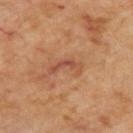Recorded during total-body skin imaging; not selected for excision or biopsy. The lesion-visualizer software estimated a border-irregularity rating of about 7/10, internal color variation of about 0 on a 0–10 scale, and peripheral color asymmetry of about 0. The analysis additionally found lesion-presence confidence of about 100/100. This image is a 15 mm lesion crop taken from a total-body photograph. The patient is roughly 65 years of age. The lesion is on the upper back. Longest diameter approximately 4 mm. The tile uses cross-polarized illumination.